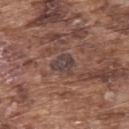workup: total-body-photography surveillance lesion; no biopsy | location: the upper back | patient: male, in their mid-70s | illumination: white-light | automated lesion analysis: an outline eccentricity of about 0.35 (0 = round, 1 = elongated) and a shape-asymmetry score of about 0.3 (0 = symmetric); an average lesion color of about L≈40 a*≈15 b*≈18 (CIELAB), a lesion–skin lightness drop of about 7, and a normalized lesion–skin contrast near 8; a border-irregularity index near 3.5/10, a within-lesion color-variation index near 3/10, and radial color variation of about 1; an automated nevus-likeness rating near 0 out of 100 and lesion-presence confidence of about 85/100 | acquisition: ~15 mm crop, total-body skin-cancer survey.